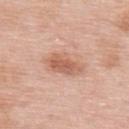Part of a total-body skin-imaging series; this lesion was reviewed on a skin check and was not flagged for biopsy.
A female patient approximately 50 years of age.
From the upper back.
The lesion-visualizer software estimated an area of roughly 9 mm² and an eccentricity of roughly 0.9. And it measured a peripheral color-asymmetry measure near 1.5.
Longest diameter approximately 5 mm.
A 15 mm crop from a total-body photograph taken for skin-cancer surveillance.